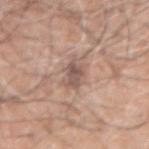The lesion was tiled from a total-body skin photograph and was not biopsied.